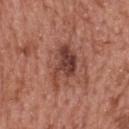biopsy status: imaged on a skin check; not biopsied
imaging modality: 15 mm crop, total-body photography
anatomic site: the upper back
image-analysis metrics: a shape eccentricity near 0.8 and a shape-asymmetry score of about 0.4 (0 = symmetric); an average lesion color of about L≈42 a*≈24 b*≈26 (CIELAB), about 12 CIELAB-L* units darker than the surrounding skin, and a normalized lesion–skin contrast near 9.5; border irregularity of about 5 on a 0–10 scale and peripheral color asymmetry of about 1.5; an automated nevus-likeness rating near 40 out of 100 and a detector confidence of about 100 out of 100 that the crop contains a lesion
lighting: white-light illumination
subject: female, aged approximately 60